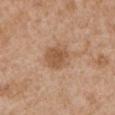Q: Is there a histopathology result?
A: total-body-photography surveillance lesion; no biopsy
Q: Who is the patient?
A: male, in their mid-60s
Q: Lesion location?
A: the left upper arm
Q: Automated lesion metrics?
A: an area of roughly 8.5 mm² and a symmetry-axis asymmetry near 0.15; roughly 9 lightness units darker than nearby skin
Q: Lesion size?
A: about 3.5 mm
Q: How was this image acquired?
A: ~15 mm crop, total-body skin-cancer survey
Q: How was the tile lit?
A: white-light illumination